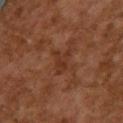{
  "biopsy_status": "not biopsied; imaged during a skin examination",
  "image": {
    "source": "total-body photography crop",
    "field_of_view_mm": 15
  },
  "automated_metrics": {
    "shape_asymmetry": 0.45,
    "peripheral_color_asymmetry": 0.5,
    "nevus_likeness_0_100": 0
  },
  "patient": {
    "sex": "female",
    "age_approx": 60
  },
  "site": "upper back"
}